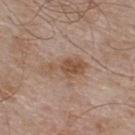Findings:
* notes: imaged on a skin check; not biopsied
* patient: male, approximately 55 years of age
* TBP lesion metrics: a footprint of about 8 mm², a shape eccentricity near 0.9, and a shape-asymmetry score of about 0.5 (0 = symmetric); border irregularity of about 5.5 on a 0–10 scale, a color-variation rating of about 3/10, and peripheral color asymmetry of about 1; an automated nevus-likeness rating near 5 out of 100
* diameter: ~5 mm (longest diameter)
* imaging modality: ~15 mm tile from a whole-body skin photo
* body site: the back